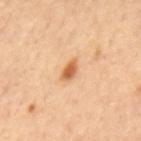Impression: The lesion was tiled from a total-body skin photograph and was not biopsied. Context: Located on the mid back. A roughly 15 mm field-of-view crop from a total-body skin photograph. A male subject, roughly 60 years of age.